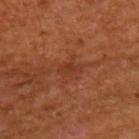This lesion was catalogued during total-body skin photography and was not selected for biopsy.
The subject is a male aged approximately 65.
The lesion is on the upper back.
A region of skin cropped from a whole-body photographic capture, roughly 15 mm wide.
Imaged with cross-polarized lighting.